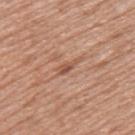Imaged with white-light lighting.
The lesion's longest dimension is about 3 mm.
Cropped from a whole-body photographic skin survey; the tile spans about 15 mm.
The lesion is on the upper back.
A female patient approximately 50 years of age.